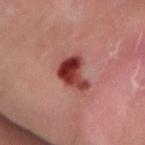Impression:
This lesion was catalogued during total-body skin photography and was not selected for biopsy.
Background:
The lesion's longest dimension is about 4 mm. A male subject approximately 65 years of age. Cropped from a whole-body photographic skin survey; the tile spans about 15 mm. Located on the right forearm.